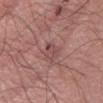Recorded during total-body skin imaging; not selected for excision or biopsy. Located on the abdomen. A 15 mm crop from a total-body photograph taken for skin-cancer surveillance. The lesion's longest dimension is about 2.5 mm. Imaged with white-light lighting. A male patient in their 70s. Automated tile analysis of the lesion measured an outline eccentricity of about 0.5 (0 = round, 1 = elongated) and two-axis asymmetry of about 0.25. The software also gave a border-irregularity rating of about 2/10 and a peripheral color-asymmetry measure near 1. The software also gave a classifier nevus-likeness of about 0/100 and lesion-presence confidence of about 100/100.